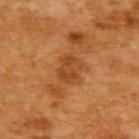follow-up — imaged on a skin check; not biopsied | anatomic site — the back | tile lighting — cross-polarized illumination | lesion diameter — ~3 mm (longest diameter) | image — 15 mm crop, total-body photography | patient — female, about 55 years old.